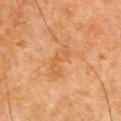No biopsy was performed on this lesion — it was imaged during a full skin examination and was not determined to be concerning. A male subject, aged around 65. The lesion's longest dimension is about 4.5 mm. The total-body-photography lesion software estimated an average lesion color of about L≈47 a*≈20 b*≈35 (CIELAB), roughly 5 lightness units darker than nearby skin, and a normalized border contrast of about 4.5. The software also gave internal color variation of about 2 on a 0–10 scale and peripheral color asymmetry of about 0.5. Cropped from a total-body skin-imaging series; the visible field is about 15 mm. From the right upper arm. Imaged with cross-polarized lighting.